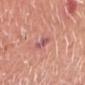Q: Was this lesion biopsied?
A: no biopsy performed (imaged during a skin exam)